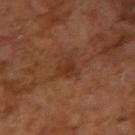Q: Was this lesion biopsied?
A: imaged on a skin check; not biopsied
Q: Who is the patient?
A: male, in their mid-50s
Q: Automated lesion metrics?
A: a mean CIELAB color near L≈33 a*≈22 b*≈30 and a normalized lesion–skin contrast near 6; a border-irregularity index near 2.5/10, a within-lesion color-variation index near 2/10, and radial color variation of about 0.5; a lesion-detection confidence of about 100/100
Q: Illumination type?
A: cross-polarized
Q: Lesion location?
A: the right upper arm
Q: Lesion size?
A: ≈2.5 mm
Q: How was this image acquired?
A: ~15 mm crop, total-body skin-cancer survey